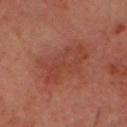Clinical impression:
Imaged during a routine full-body skin examination; the lesion was not biopsied and no histopathology is available.
Background:
Approximately 6 mm at its widest. The subject is a male aged around 40. Automated image analysis of the tile measured a border-irregularity index near 3.5/10 and radial color variation of about 1. And it measured lesion-presence confidence of about 100/100. A lesion tile, about 15 mm wide, cut from a 3D total-body photograph. On the head or neck.